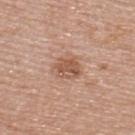Impression:
This lesion was catalogued during total-body skin photography and was not selected for biopsy.
Background:
A female subject roughly 50 years of age. Captured under white-light illumination. A lesion tile, about 15 mm wide, cut from a 3D total-body photograph. The lesion is located on the back. Longest diameter approximately 3.5 mm.